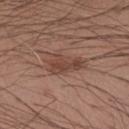  biopsy_status: not biopsied; imaged during a skin examination
  automated_metrics:
    cielab_L: 44
    cielab_a: 20
    cielab_b: 25
    vs_skin_darker_L: 8.0
  site: left forearm
  patient:
    sex: male
    age_approx: 30
  lesion_size:
    long_diameter_mm_approx: 4.5
  lighting: white-light
  image:
    source: total-body photography crop
    field_of_view_mm: 15